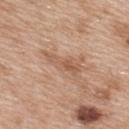This lesion was catalogued during total-body skin photography and was not selected for biopsy.
Captured under white-light illumination.
A female patient in their 40s.
A region of skin cropped from a whole-body photographic capture, roughly 15 mm wide.
An algorithmic analysis of the crop reported an average lesion color of about L≈57 a*≈20 b*≈31 (CIELAB), a lesion–skin lightness drop of about 8, and a normalized lesion–skin contrast near 6. It also reported border irregularity of about 5.5 on a 0–10 scale, a within-lesion color-variation index near 3/10, and a peripheral color-asymmetry measure near 1. The software also gave an automated nevus-likeness rating near 0 out of 100 and lesion-presence confidence of about 95/100.
Measured at roughly 5 mm in maximum diameter.
The lesion is on the upper back.